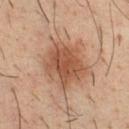Recorded during total-body skin imaging; not selected for excision or biopsy.
Longest diameter approximately 5.5 mm.
The tile uses cross-polarized illumination.
The lesion is on the chest.
Cropped from a whole-body photographic skin survey; the tile spans about 15 mm.
The patient is a male in their mid-30s.